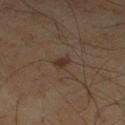Clinical impression:
The lesion was tiled from a total-body skin photograph and was not biopsied.
Background:
Automated image analysis of the tile measured a mean CIELAB color near L≈30 a*≈12 b*≈22, about 5 CIELAB-L* units darker than the surrounding skin, and a lesion-to-skin contrast of about 6 (normalized; higher = more distinct). The analysis additionally found a lesion-detection confidence of about 100/100. The recorded lesion diameter is about 2.5 mm. The lesion is on the leg. A 15 mm close-up extracted from a 3D total-body photography capture. This is a cross-polarized tile. The patient is a male aged 58–62.